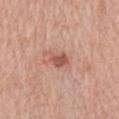Clinical impression:
No biopsy was performed on this lesion — it was imaged during a full skin examination and was not determined to be concerning.
Image and clinical context:
A 15 mm crop from a total-body photograph taken for skin-cancer surveillance. A male subject, in their 80s. About 3 mm across. From the right upper arm.